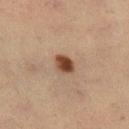No biopsy was performed on this lesion — it was imaged during a full skin examination and was not determined to be concerning. The subject is a female aged 53 to 57. Imaged with cross-polarized lighting. About 3 mm across. A 15 mm close-up extracted from a 3D total-body photography capture. Located on the right lower leg.